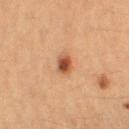notes = catalogued during a skin exam; not biopsied | site = the left lower leg | subject = female, aged 38–42 | lighting = cross-polarized illumination | automated metrics = an automated nevus-likeness rating near 100 out of 100 and a lesion-detection confidence of about 100/100 | acquisition = 15 mm crop, total-body photography | lesion diameter = about 2.5 mm.